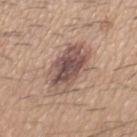<tbp_lesion>
<biopsy_status>not biopsied; imaged during a skin examination</biopsy_status>
<image>
  <source>total-body photography crop</source>
  <field_of_view_mm>15</field_of_view_mm>
</image>
<patient>
  <sex>male</sex>
  <age_approx>60</age_approx>
</patient>
<site>mid back</site>
<automated_metrics>
  <area_mm2_approx>21.0</area_mm2_approx>
  <cielab_L>53</cielab_L>
  <cielab_a>17</cielab_a>
  <cielab_b>23</cielab_b>
  <vs_skin_darker_L>13.0</vs_skin_darker_L>
  <vs_skin_contrast_norm>9.0</vs_skin_contrast_norm>
  <nevus_likeness_0_100>40</nevus_likeness_0_100>
  <lesion_detection_confidence_0_100>100</lesion_detection_confidence_0_100>
</automated_metrics>
<lesion_size>
  <long_diameter_mm_approx>7.0</long_diameter_mm_approx>
</lesion_size>
<lighting>white-light</lighting>
</tbp_lesion>